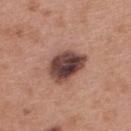Impression: This lesion was catalogued during total-body skin photography and was not selected for biopsy. Image and clinical context: From the upper back. Imaged with white-light lighting. A 15 mm crop from a total-body photograph taken for skin-cancer surveillance. About 5 mm across. The subject is a female about 40 years old.